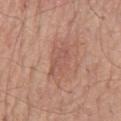Notes:
• notes · catalogued during a skin exam; not biopsied
• patient · male, aged 68–72
• tile lighting · white-light illumination
• diameter · ≈4.5 mm
• location · the mid back
• image-analysis metrics · a footprint of about 8 mm², a shape eccentricity near 0.8, and two-axis asymmetry of about 0.3; a border-irregularity rating of about 4.5/10, internal color variation of about 2 on a 0–10 scale, and radial color variation of about 1; a lesion-detection confidence of about 100/100
• image source · ~15 mm tile from a whole-body skin photo